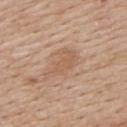<tbp_lesion>
<biopsy_status>not biopsied; imaged during a skin examination</biopsy_status>
<site>upper back</site>
<image>
  <source>total-body photography crop</source>
  <field_of_view_mm>15</field_of_view_mm>
</image>
<lesion_size>
  <long_diameter_mm_approx>4.5</long_diameter_mm_approx>
</lesion_size>
<automated_metrics>
  <cielab_L>58</cielab_L>
  <cielab_a>19</cielab_a>
  <cielab_b>31</cielab_b>
  <vs_skin_darker_L>7.0</vs_skin_darker_L>
  <vs_skin_contrast_norm>5.0</vs_skin_contrast_norm>
  <border_irregularity_0_10>5.5</border_irregularity_0_10>
  <peripheral_color_asymmetry>0.5</peripheral_color_asymmetry>
  <nevus_likeness_0_100>10</nevus_likeness_0_100>
  <lesion_detection_confidence_0_100>100</lesion_detection_confidence_0_100>
</automated_metrics>
<lighting>white-light</lighting>
<patient>
  <sex>male</sex>
  <age_approx>60</age_approx>
</patient>
</tbp_lesion>